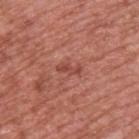Part of a total-body skin-imaging series; this lesion was reviewed on a skin check and was not flagged for biopsy.